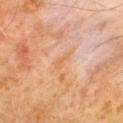{"biopsy_status": "not biopsied; imaged during a skin examination", "image": {"source": "total-body photography crop", "field_of_view_mm": 15}, "patient": {"sex": "male", "age_approx": 60}, "site": "chest"}